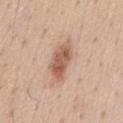Recorded during total-body skin imaging; not selected for excision or biopsy.
A male subject aged around 45.
Located on the chest.
A lesion tile, about 15 mm wide, cut from a 3D total-body photograph.
Captured under white-light illumination.